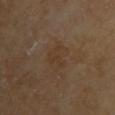<lesion>
<biopsy_status>not biopsied; imaged during a skin examination</biopsy_status>
<lesion_size>
  <long_diameter_mm_approx>3.5</long_diameter_mm_approx>
</lesion_size>
<patient>
  <sex>female</sex>
  <age_approx>70</age_approx>
</patient>
<automated_metrics>
  <area_mm2_approx>6.5</area_mm2_approx>
  <eccentricity>0.75</eccentricity>
  <cielab_L>36</cielab_L>
  <cielab_a>15</cielab_a>
  <cielab_b>28</cielab_b>
  <vs_skin_darker_L>4.0</vs_skin_darker_L>
  <vs_skin_contrast_norm>5.0</vs_skin_contrast_norm>
  <nevus_likeness_0_100>0</nevus_likeness_0_100>
  <lesion_detection_confidence_0_100>100</lesion_detection_confidence_0_100>
</automated_metrics>
<lighting>cross-polarized</lighting>
<image>
  <source>total-body photography crop</source>
  <field_of_view_mm>15</field_of_view_mm>
</image>
<site>upper back</site>
</lesion>